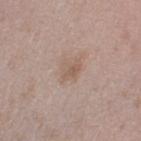{"biopsy_status": "not biopsied; imaged during a skin examination", "site": "arm", "patient": {"sex": "male", "age_approx": 50}, "lighting": "white-light", "lesion_size": {"long_diameter_mm_approx": 2.5}, "image": {"source": "total-body photography crop", "field_of_view_mm": 15}, "automated_metrics": {"area_mm2_approx": 4.5, "shape_asymmetry": 0.25, "cielab_L": 57, "cielab_a": 16, "cielab_b": 26, "vs_skin_darker_L": 7.0, "nevus_likeness_0_100": 5, "lesion_detection_confidence_0_100": 100}}